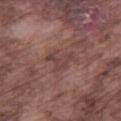No biopsy was performed on this lesion — it was imaged during a full skin examination and was not determined to be concerning.
The subject is a male about 75 years old.
Located on the right thigh.
A lesion tile, about 15 mm wide, cut from a 3D total-body photograph.
The lesion's longest dimension is about 3.5 mm.